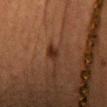Image and clinical context: A female patient aged approximately 40. Located on the head or neck. Measured at roughly 2.5 mm in maximum diameter. A 15 mm close-up tile from a total-body photography series done for melanoma screening. Captured under cross-polarized illumination.